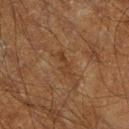Assessment: The lesion was photographed on a routine skin check and not biopsied; there is no pathology result. Context: A male subject, approximately 60 years of age. Located on the right lower leg. A roughly 15 mm field-of-view crop from a total-body skin photograph.